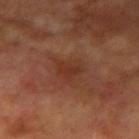Image and clinical context:
This image is a 15 mm lesion crop taken from a total-body photograph. The patient is a male approximately 70 years of age. Located on the right upper arm.